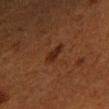A region of skin cropped from a whole-body photographic capture, roughly 15 mm wide.
Measured at roughly 2.5 mm in maximum diameter.
A female subject, aged around 55.
The lesion is located on the arm.
Automated image analysis of the tile measured an area of roughly 3.5 mm², an outline eccentricity of about 0.8 (0 = round, 1 = elongated), and a shape-asymmetry score of about 0.35 (0 = symmetric). It also reported a mean CIELAB color near L≈22 a*≈19 b*≈26 and a normalized border contrast of about 8. The software also gave border irregularity of about 3 on a 0–10 scale and radial color variation of about 0.